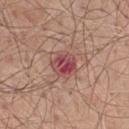workup — imaged on a skin check; not biopsied | body site — the front of the torso | subject — male, about 50 years old | acquisition — ~15 mm crop, total-body skin-cancer survey | lesion size — ≈3 mm | illumination — white-light illumination.